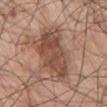Part of a total-body skin-imaging series; this lesion was reviewed on a skin check and was not flagged for biopsy. The patient is a male aged approximately 70. Approximately 7.5 mm at its widest. A lesion tile, about 15 mm wide, cut from a 3D total-body photograph. The total-body-photography lesion software estimated a mean CIELAB color near L≈49 a*≈20 b*≈28, roughly 11 lightness units darker than nearby skin, and a lesion-to-skin contrast of about 8 (normalized; higher = more distinct). It also reported an automated nevus-likeness rating near 40 out of 100 and lesion-presence confidence of about 100/100. This is a white-light tile. From the chest.